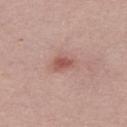Q: Is there a histopathology result?
A: no biopsy performed (imaged during a skin exam)
Q: How was the tile lit?
A: white-light illumination
Q: What are the patient's age and sex?
A: male, about 30 years old
Q: Automated lesion metrics?
A: a lesion area of about 4.5 mm², a shape eccentricity near 0.8, and two-axis asymmetry of about 0.3; a classifier nevus-likeness of about 25/100
Q: How large is the lesion?
A: about 3 mm
Q: What is the imaging modality?
A: total-body-photography crop, ~15 mm field of view